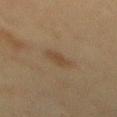Assessment: Recorded during total-body skin imaging; not selected for excision or biopsy. Background: The lesion is on the mid back. A 15 mm close-up tile from a total-body photography series done for melanoma screening. The subject is a female aged 53 to 57.